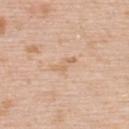Impression:
This lesion was catalogued during total-body skin photography and was not selected for biopsy.
Image and clinical context:
The lesion is located on the upper back. A 15 mm close-up tile from a total-body photography series done for melanoma screening. The patient is a male aged approximately 60.